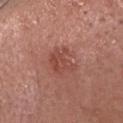Recorded during total-body skin imaging; not selected for excision or biopsy.
A roughly 15 mm field-of-view crop from a total-body skin photograph.
On the head or neck.
An algorithmic analysis of the crop reported a lesion area of about 6.5 mm², an outline eccentricity of about 0.5 (0 = round, 1 = elongated), and a shape-asymmetry score of about 0.35 (0 = symmetric). The software also gave an average lesion color of about L≈47 a*≈27 b*≈28 (CIELAB), roughly 8 lightness units darker than nearby skin, and a lesion-to-skin contrast of about 6 (normalized; higher = more distinct). It also reported a nevus-likeness score of about 25/100 and a detector confidence of about 100 out of 100 that the crop contains a lesion.
This is a white-light tile.
A female subject, aged around 65.
Longest diameter approximately 3 mm.